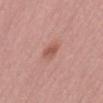Notes:
• biopsy status — no biopsy performed (imaged during a skin exam)
• lesion size — ≈2.5 mm
• body site — the front of the torso
• automated lesion analysis — a lesion area of about 4 mm²; a lesion color around L≈55 a*≈25 b*≈27 in CIELAB, about 9 CIELAB-L* units darker than the surrounding skin, and a lesion-to-skin contrast of about 6.5 (normalized; higher = more distinct); an automated nevus-likeness rating near 75 out of 100 and a lesion-detection confidence of about 100/100
• acquisition — ~15 mm tile from a whole-body skin photo
• illumination — white-light
• patient — female, approximately 70 years of age